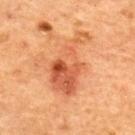Captured during whole-body skin photography for melanoma surveillance; the lesion was not biopsied.
The patient is a female aged 68–72.
This image is a 15 mm lesion crop taken from a total-body photograph.
Longest diameter approximately 7.5 mm.
Captured under cross-polarized illumination.
From the upper back.